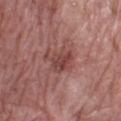Q: Was this lesion biopsied?
A: catalogued during a skin exam; not biopsied
Q: How was this image acquired?
A: ~15 mm tile from a whole-body skin photo
Q: Illumination type?
A: white-light illumination
Q: Where on the body is the lesion?
A: the right lower leg
Q: Who is the patient?
A: female, about 70 years old
Q: What did automated image analysis measure?
A: an outline eccentricity of about 0.7 (0 = round, 1 = elongated) and a shape-asymmetry score of about 0.35 (0 = symmetric); a lesion color around L≈44 a*≈25 b*≈23 in CIELAB, a lesion–skin lightness drop of about 9, and a lesion-to-skin contrast of about 7 (normalized; higher = more distinct)
Q: What is the lesion's diameter?
A: ≈4 mm